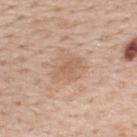Findings:
* biopsy status · no biopsy performed (imaged during a skin exam)
* site · the upper back
* acquisition · ~15 mm tile from a whole-body skin photo
* subject · male, aged around 60
* tile lighting · white-light